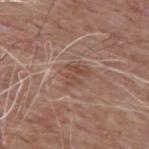follow-up = total-body-photography surveillance lesion; no biopsy
image-analysis metrics = a lesion color around L≈48 a*≈19 b*≈26 in CIELAB and a lesion–skin lightness drop of about 7; an automated nevus-likeness rating near 0 out of 100 and lesion-presence confidence of about 100/100
subject = male, roughly 60 years of age
image = ~15 mm tile from a whole-body skin photo
lesion size = about 3.5 mm
body site = the mid back